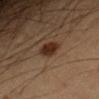Imaged during a routine full-body skin examination; the lesion was not biopsied and no histopathology is available. A male subject aged approximately 55. A 15 mm crop from a total-body photograph taken for skin-cancer surveillance. The recorded lesion diameter is about 3.5 mm. Located on the left forearm. The tile uses cross-polarized illumination.